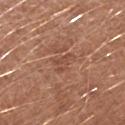Notes:
* follow-up: catalogued during a skin exam; not biopsied
* anatomic site: the left forearm
* subject: female, roughly 40 years of age
* lesion size: ≈3 mm
* image: 15 mm crop, total-body photography
* tile lighting: white-light illumination